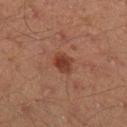{
  "biopsy_status": "not biopsied; imaged during a skin examination",
  "site": "right lower leg",
  "image": {
    "source": "total-body photography crop",
    "field_of_view_mm": 15
  },
  "lighting": "cross-polarized",
  "patient": {
    "sex": "male",
    "age_approx": 45
  }
}